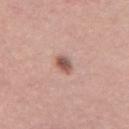diameter: ≈2.5 mm | patient: male, aged approximately 40 | image source: ~15 mm tile from a whole-body skin photo | site: the mid back | automated metrics: a border-irregularity index near 2/10 and a within-lesion color-variation index near 4/10 | illumination: white-light.